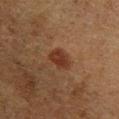Impression:
No biopsy was performed on this lesion — it was imaged during a full skin examination and was not determined to be concerning.
Background:
A female patient roughly 50 years of age. The lesion-visualizer software estimated a classifier nevus-likeness of about 95/100 and a lesion-detection confidence of about 100/100. Cropped from a whole-body photographic skin survey; the tile spans about 15 mm. From the leg. Approximately 3 mm at its widest.